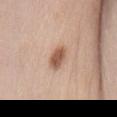| key | value |
|---|---|
| biopsy status | total-body-photography surveillance lesion; no biopsy |
| diameter | ≈3 mm |
| site | the abdomen |
| image source | total-body-photography crop, ~15 mm field of view |
| TBP lesion metrics | a lesion area of about 5.5 mm², a shape eccentricity near 0.8, and two-axis asymmetry of about 0.15; roughly 13 lightness units darker than nearby skin and a normalized lesion–skin contrast near 9; a nevus-likeness score of about 95/100 and lesion-presence confidence of about 100/100 |
| subject | female, aged approximately 50 |